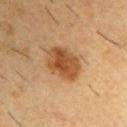The lesion was tiled from a total-body skin photograph and was not biopsied. The lesion is on the upper back. About 4.5 mm across. Cropped from a total-body skin-imaging series; the visible field is about 15 mm. A male subject, aged 53 to 57. Automated tile analysis of the lesion measured an area of roughly 13 mm² and a symmetry-axis asymmetry near 0.15. It also reported a border-irregularity rating of about 1.5/10 and internal color variation of about 4.5 on a 0–10 scale. This is a cross-polarized tile.